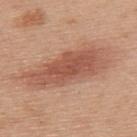Captured during whole-body skin photography for melanoma surveillance; the lesion was not biopsied.
This is a white-light tile.
An algorithmic analysis of the crop reported two-axis asymmetry of about 0.25. The software also gave an average lesion color of about L≈54 a*≈24 b*≈30 (CIELAB), about 11 CIELAB-L* units darker than the surrounding skin, and a normalized border contrast of about 7.5. The software also gave a border-irregularity rating of about 4/10.
Located on the upper back.
The patient is a male aged around 70.
Measured at roughly 9.5 mm in maximum diameter.
A close-up tile cropped from a whole-body skin photograph, about 15 mm across.